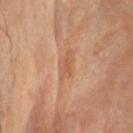Part of a total-body skin-imaging series; this lesion was reviewed on a skin check and was not flagged for biopsy. An algorithmic analysis of the crop reported a footprint of about 5.5 mm², an outline eccentricity of about 0.9 (0 = round, 1 = elongated), and a shape-asymmetry score of about 0.3 (0 = symmetric). And it measured an automated nevus-likeness rating near 0 out of 100 and a lesion-detection confidence of about 95/100. Approximately 4 mm at its widest. Located on the head or neck. This is a cross-polarized tile. This image is a 15 mm lesion crop taken from a total-body photograph.